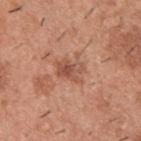The lesion was photographed on a routine skin check and not biopsied; there is no pathology result.
Located on the upper back.
An algorithmic analysis of the crop reported an average lesion color of about L≈53 a*≈24 b*≈30 (CIELAB), roughly 9 lightness units darker than nearby skin, and a normalized border contrast of about 6.5. It also reported peripheral color asymmetry of about 2. The analysis additionally found a nevus-likeness score of about 5/100 and a lesion-detection confidence of about 100/100.
The patient is a male in their 40s.
The tile uses white-light illumination.
Measured at roughly 3.5 mm in maximum diameter.
A close-up tile cropped from a whole-body skin photograph, about 15 mm across.